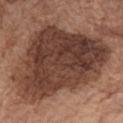Clinical impression: This lesion was catalogued during total-body skin photography and was not selected for biopsy. Acquisition and patient details: The lesion is on the chest. This is a white-light tile. A male subject aged around 75. A 15 mm close-up tile from a total-body photography series done for melanoma screening. The lesion-visualizer software estimated a lesion color around L≈38 a*≈19 b*≈25 in CIELAB, about 14 CIELAB-L* units darker than the surrounding skin, and a normalized lesion–skin contrast near 11.5. And it measured a border-irregularity index near 4/10, a color-variation rating of about 5.5/10, and radial color variation of about 2. The recorded lesion diameter is about 11.5 mm.